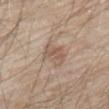The lesion was tiled from a total-body skin photograph and was not biopsied. A 15 mm crop from a total-body photograph taken for skin-cancer surveillance. A male patient, roughly 80 years of age. This is a white-light tile. On the abdomen.